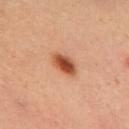Assessment:
Imaged during a routine full-body skin examination; the lesion was not biopsied and no histopathology is available.
Background:
The lesion is on the upper back. A lesion tile, about 15 mm wide, cut from a 3D total-body photograph. Approximately 3.5 mm at its widest. The subject is a female aged approximately 40. Automated image analysis of the tile measured about 16 CIELAB-L* units darker than the surrounding skin and a normalized border contrast of about 10.5. It also reported a border-irregularity rating of about 1.5/10. And it measured an automated nevus-likeness rating near 100 out of 100 and lesion-presence confidence of about 100/100.